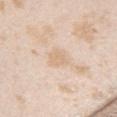follow-up — catalogued during a skin exam; not biopsied
patient — female, aged around 25
lighting — white-light illumination
site — the back
image — total-body-photography crop, ~15 mm field of view